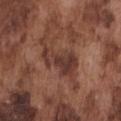Clinical impression:
Recorded during total-body skin imaging; not selected for excision or biopsy.
Acquisition and patient details:
The recorded lesion diameter is about 6.5 mm. The total-body-photography lesion software estimated a lesion area of about 12 mm² and an eccentricity of roughly 0.9. The analysis additionally found border irregularity of about 7 on a 0–10 scale and internal color variation of about 5 on a 0–10 scale. It also reported a detector confidence of about 95 out of 100 that the crop contains a lesion. A male subject aged 73–77. Cropped from a whole-body photographic skin survey; the tile spans about 15 mm. The lesion is located on the chest. Captured under white-light illumination.